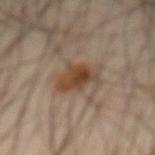- follow-up: catalogued during a skin exam; not biopsied
- site: the abdomen
- subject: male, approximately 60 years of age
- lesion diameter: ~4 mm (longest diameter)
- tile lighting: cross-polarized illumination
- image: total-body-photography crop, ~15 mm field of view